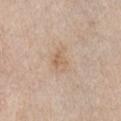<lesion>
<biopsy_status>not biopsied; imaged during a skin examination</biopsy_status>
<patient>
  <sex>male</sex>
  <age_approx>75</age_approx>
</patient>
<image>
  <source>total-body photography crop</source>
  <field_of_view_mm>15</field_of_view_mm>
</image>
<site>arm</site>
</lesion>